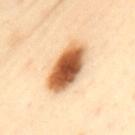Recorded during total-body skin imaging; not selected for excision or biopsy.
A close-up tile cropped from a whole-body skin photograph, about 15 mm across.
Longest diameter approximately 6.5 mm.
Automated image analysis of the tile measured a footprint of about 18 mm², an eccentricity of roughly 0.85, and two-axis asymmetry of about 0.15. And it measured a border-irregularity index near 2/10 and internal color variation of about 8.5 on a 0–10 scale. The software also gave a classifier nevus-likeness of about 100/100 and lesion-presence confidence of about 100/100.
The subject is a female about 40 years old.
This is a cross-polarized tile.
From the upper back.